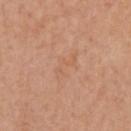<record>
<biopsy_status>not biopsied; imaged during a skin examination</biopsy_status>
<lesion_size>
  <long_diameter_mm_approx>3.5</long_diameter_mm_approx>
</lesion_size>
<site>left upper arm</site>
<patient>
  <sex>female</sex>
  <age_approx>45</age_approx>
</patient>
<image>
  <source>total-body photography crop</source>
  <field_of_view_mm>15</field_of_view_mm>
</image>
<lighting>white-light</lighting>
</record>